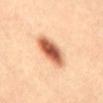Impression:
Captured during whole-body skin photography for melanoma surveillance; the lesion was not biopsied.
Clinical summary:
Automated tile analysis of the lesion measured a mean CIELAB color near L≈58 a*≈26 b*≈35, a lesion–skin lightness drop of about 20, and a normalized lesion–skin contrast near 11.5. The software also gave border irregularity of about 2 on a 0–10 scale and peripheral color asymmetry of about 2. The analysis additionally found a classifier nevus-likeness of about 100/100 and lesion-presence confidence of about 100/100. Imaged with cross-polarized lighting. On the chest. The lesion's longest dimension is about 5 mm. A male subject, aged approximately 20. Cropped from a whole-body photographic skin survey; the tile spans about 15 mm.